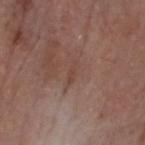Clinical impression: No biopsy was performed on this lesion — it was imaged during a full skin examination and was not determined to be concerning. Clinical summary: A male subject, aged 78 to 82. The lesion is on the head or neck. A 15 mm crop from a total-body photograph taken for skin-cancer surveillance. An algorithmic analysis of the crop reported a mean CIELAB color near L≈44 a*≈20 b*≈24, a lesion–skin lightness drop of about 5, and a normalized lesion–skin contrast near 4.5. The analysis additionally found internal color variation of about 0 on a 0–10 scale and a peripheral color-asymmetry measure near 0. It also reported a lesion-detection confidence of about 85/100. Captured under white-light illumination. Measured at roughly 2.5 mm in maximum diameter.